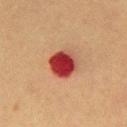Impression:
The lesion was photographed on a routine skin check and not biopsied; there is no pathology result.
Clinical summary:
The lesion is located on the mid back. The tile uses cross-polarized illumination. A male patient aged approximately 40. The recorded lesion diameter is about 4 mm. Automated image analysis of the tile measured a mean CIELAB color near L≈35 a*≈33 b*≈27, a lesion–skin lightness drop of about 17, and a lesion-to-skin contrast of about 14 (normalized; higher = more distinct). The software also gave a within-lesion color-variation index near 7/10 and peripheral color asymmetry of about 2.5. And it measured a classifier nevus-likeness of about 0/100 and a detector confidence of about 100 out of 100 that the crop contains a lesion. A lesion tile, about 15 mm wide, cut from a 3D total-body photograph.